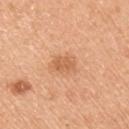Recorded during total-body skin imaging; not selected for excision or biopsy.
A 15 mm close-up extracted from a 3D total-body photography capture.
The patient is a male aged around 50.
The lesion-visualizer software estimated a footprint of about 5.5 mm², an outline eccentricity of about 0.7 (0 = round, 1 = elongated), and a shape-asymmetry score of about 0.2 (0 = symmetric). It also reported a border-irregularity rating of about 2.5/10 and radial color variation of about 0.5.
The recorded lesion diameter is about 3 mm.
On the right upper arm.
Captured under white-light illumination.